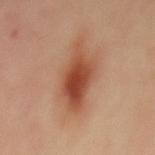| feature | finding |
|---|---|
| workup | total-body-photography surveillance lesion; no biopsy |
| illumination | cross-polarized |
| subject | female, roughly 35 years of age |
| image source | ~15 mm tile from a whole-body skin photo |
| diameter | about 8 mm |
| anatomic site | the mid back |